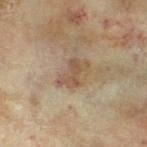Case summary:
• tile lighting · cross-polarized
• body site · the leg
• image source · total-body-photography crop, ~15 mm field of view
• diameter · about 3.5 mm
• automated metrics · an area of roughly 6.5 mm², a shape eccentricity near 0.8, and a symmetry-axis asymmetry near 0.4; a lesion color around L≈47 a*≈14 b*≈27 in CIELAB, a lesion–skin lightness drop of about 8, and a normalized lesion–skin contrast near 6; a classifier nevus-likeness of about 0/100 and a lesion-detection confidence of about 100/100
• subject · female, aged 58–62